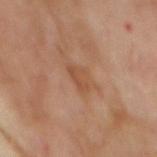No biopsy was performed on this lesion — it was imaged during a full skin examination and was not determined to be concerning.